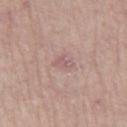Q: Is there a histopathology result?
A: catalogued during a skin exam; not biopsied
Q: Lesion size?
A: ~2.5 mm (longest diameter)
Q: Where on the body is the lesion?
A: the right thigh
Q: How was this image acquired?
A: ~15 mm tile from a whole-body skin photo
Q: What are the patient's age and sex?
A: female, aged 68 to 72
Q: Automated lesion metrics?
A: a lesion color around L≈58 a*≈20 b*≈19 in CIELAB, roughly 7 lightness units darker than nearby skin, and a normalized border contrast of about 5; radial color variation of about 0.5
Q: Illumination type?
A: white-light